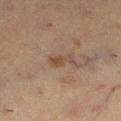The lesion was tiled from a total-body skin photograph and was not biopsied.
A female subject aged 38–42.
The lesion is located on the left lower leg.
A close-up tile cropped from a whole-body skin photograph, about 15 mm across.